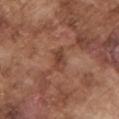Q: Was this lesion biopsied?
A: no biopsy performed (imaged during a skin exam)
Q: What are the patient's age and sex?
A: male, aged 73 to 77
Q: How large is the lesion?
A: ~3 mm (longest diameter)
Q: What kind of image is this?
A: total-body-photography crop, ~15 mm field of view
Q: What did automated image analysis measure?
A: a mean CIELAB color near L≈42 a*≈22 b*≈28, roughly 8 lightness units darker than nearby skin, and a normalized border contrast of about 6.5; internal color variation of about 2.5 on a 0–10 scale and peripheral color asymmetry of about 1; an automated nevus-likeness rating near 0 out of 100 and a lesion-detection confidence of about 95/100
Q: Lesion location?
A: the left upper arm
Q: Illumination type?
A: white-light illumination